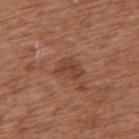Q: Is there a histopathology result?
A: catalogued during a skin exam; not biopsied
Q: What is the anatomic site?
A: the upper back
Q: Illumination type?
A: white-light illumination
Q: What are the patient's age and sex?
A: female, aged 73–77
Q: What is the imaging modality?
A: ~15 mm crop, total-body skin-cancer survey
Q: What did automated image analysis measure?
A: a lesion color around L≈42 a*≈24 b*≈29 in CIELAB, a lesion–skin lightness drop of about 8, and a lesion-to-skin contrast of about 6.5 (normalized; higher = more distinct); lesion-presence confidence of about 100/100
Q: What is the lesion's diameter?
A: about 3 mm